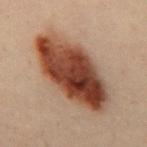Assessment:
Part of a total-body skin-imaging series; this lesion was reviewed on a skin check and was not flagged for biopsy.
Background:
A 15 mm crop from a total-body photograph taken for skin-cancer surveillance. Automated tile analysis of the lesion measured about 16 CIELAB-L* units darker than the surrounding skin and a normalized border contrast of about 13.5. It also reported border irregularity of about 2 on a 0–10 scale, a color-variation rating of about 9.5/10, and peripheral color asymmetry of about 3.5. It also reported a classifier nevus-likeness of about 95/100 and a lesion-detection confidence of about 100/100. The subject is a male in their 50s. The tile uses cross-polarized illumination. Located on the back.